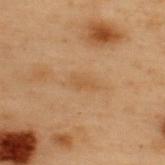| key | value |
|---|---|
| workup | no biopsy performed (imaged during a skin exam) |
| lesion size | ~2.5 mm (longest diameter) |
| image-analysis metrics | a lesion–skin lightness drop of about 5 and a normalized lesion–skin contrast near 5; peripheral color asymmetry of about 0 |
| image source | total-body-photography crop, ~15 mm field of view |
| tile lighting | cross-polarized |
| body site | the upper back |
| patient | male, aged 53–57 |